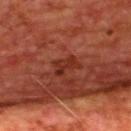Q: Is there a histopathology result?
A: total-body-photography surveillance lesion; no biopsy
Q: Who is the patient?
A: male, roughly 65 years of age
Q: What did automated image analysis measure?
A: an area of roughly 4.5 mm², an eccentricity of roughly 0.75, and a shape-asymmetry score of about 0.35 (0 = symmetric)
Q: Illumination type?
A: cross-polarized
Q: What is the anatomic site?
A: the upper back
Q: How was this image acquired?
A: total-body-photography crop, ~15 mm field of view
Q: What is the lesion's diameter?
A: about 3 mm